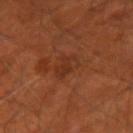The lesion was photographed on a routine skin check and not biopsied; there is no pathology result.
A lesion tile, about 15 mm wide, cut from a 3D total-body photograph.
Located on the left arm.
A male patient aged around 50.